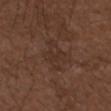Notes:
* workup — catalogued during a skin exam; not biopsied